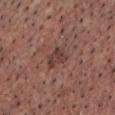Assessment: Imaged during a routine full-body skin examination; the lesion was not biopsied and no histopathology is available. Acquisition and patient details: Located on the chest. Measured at roughly 3 mm in maximum diameter. Cropped from a whole-body photographic skin survey; the tile spans about 15 mm. A male patient, aged 53–57. The tile uses white-light illumination.